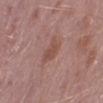biopsy status: total-body-photography surveillance lesion; no biopsy
site: the right lower leg
tile lighting: white-light illumination
image: total-body-photography crop, ~15 mm field of view
patient: male, aged 38 to 42
TBP lesion metrics: an area of roughly 5 mm² and an outline eccentricity of about 0.7 (0 = round, 1 = elongated)